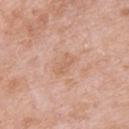Clinical impression: Imaged during a routine full-body skin examination; the lesion was not biopsied and no histopathology is available. Acquisition and patient details: A 15 mm crop from a total-body photograph taken for skin-cancer surveillance. Automated image analysis of the tile measured a lesion area of about 5 mm². The analysis additionally found a border-irregularity index near 2.5/10, a within-lesion color-variation index near 2/10, and radial color variation of about 0.5. About 3 mm across. The tile uses white-light illumination. On the back. The subject is a female about 65 years old.